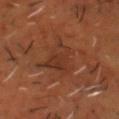The lesion was photographed on a routine skin check and not biopsied; there is no pathology result. This is a cross-polarized tile. Located on the head or neck. A male subject, approximately 50 years of age. A 15 mm crop from a total-body photograph taken for skin-cancer surveillance.